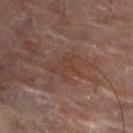Findings:
• biopsy status: no biopsy performed (imaged during a skin exam)
• acquisition: ~15 mm crop, total-body skin-cancer survey
• patient: female, aged 78–82
• lesion diameter: about 4.5 mm
• location: the right leg
• lighting: cross-polarized